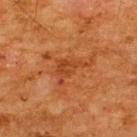The lesion was tiled from a total-body skin photograph and was not biopsied.